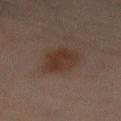Assessment: The lesion was tiled from a total-body skin photograph and was not biopsied. Image and clinical context: Cropped from a total-body skin-imaging series; the visible field is about 15 mm. On the lower back. Longest diameter approximately 5 mm. A male patient, in their mid-40s.